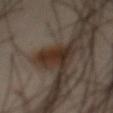{"biopsy_status": "not biopsied; imaged during a skin examination", "lighting": "cross-polarized", "site": "abdomen", "patient": {"sex": "male", "age_approx": 45}, "lesion_size": {"long_diameter_mm_approx": 4.5}, "automated_metrics": {"area_mm2_approx": 8.0, "shape_asymmetry": 0.35, "cielab_L": 28, "cielab_a": 14, "cielab_b": 22, "vs_skin_contrast_norm": 11.0}, "image": {"source": "total-body photography crop", "field_of_view_mm": 15}}